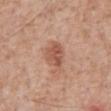Notes:
- follow-up: catalogued during a skin exam; not biopsied
- patient: male, aged approximately 60
- anatomic site: the abdomen
- acquisition: ~15 mm tile from a whole-body skin photo
- lighting: white-light illumination
- size: about 4 mm
- TBP lesion metrics: an area of roughly 8 mm² and an eccentricity of roughly 0.7; an average lesion color of about L≈55 a*≈23 b*≈30 (CIELAB), a lesion–skin lightness drop of about 10, and a normalized border contrast of about 7; internal color variation of about 3.5 on a 0–10 scale and a peripheral color-asymmetry measure near 1